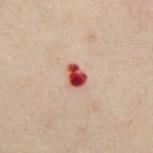{"site": "chest", "lesion_size": {"long_diameter_mm_approx": 2.5}, "automated_metrics": {"eccentricity": 0.7, "shape_asymmetry": 0.25, "cielab_L": 40, "cielab_a": 31, "cielab_b": 25, "vs_skin_darker_L": 18.0, "border_irregularity_0_10": 2.0, "peripheral_color_asymmetry": 2.0, "nevus_likeness_0_100": 0}, "image": {"source": "total-body photography crop", "field_of_view_mm": 15}, "patient": {"sex": "male", "age_approx": 50}}